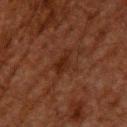Assessment:
Part of a total-body skin-imaging series; this lesion was reviewed on a skin check and was not flagged for biopsy.
Image and clinical context:
A male patient, aged 58 to 62. The lesion is on the upper back. A region of skin cropped from a whole-body photographic capture, roughly 15 mm wide. The recorded lesion diameter is about 3 mm. Imaged with cross-polarized lighting.